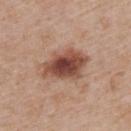Impression:
The lesion was tiled from a total-body skin photograph and was not biopsied.
Background:
A roughly 15 mm field-of-view crop from a total-body skin photograph. On the upper back. Longest diameter approximately 6 mm. Automated tile analysis of the lesion measured a footprint of about 14 mm² and an outline eccentricity of about 0.8 (0 = round, 1 = elongated). The software also gave a classifier nevus-likeness of about 95/100 and a detector confidence of about 100 out of 100 that the crop contains a lesion. A male subject approximately 75 years of age. The tile uses white-light illumination.